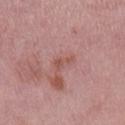Q: Was this lesion biopsied?
A: total-body-photography surveillance lesion; no biopsy
Q: Lesion location?
A: the right lower leg
Q: Automated lesion metrics?
A: an average lesion color of about L≈53 a*≈24 b*≈24 (CIELAB), roughly 7 lightness units darker than nearby skin, and a normalized border contrast of about 6
Q: What are the patient's age and sex?
A: female, in their 50s
Q: Lesion size?
A: ≈3 mm
Q: How was this image acquired?
A: ~15 mm tile from a whole-body skin photo